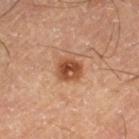The lesion was photographed on a routine skin check and not biopsied; there is no pathology result.
A lesion tile, about 15 mm wide, cut from a 3D total-body photograph.
The total-body-photography lesion software estimated a footprint of about 6.5 mm² and two-axis asymmetry of about 0.15. It also reported a border-irregularity rating of about 1.5/10 and a peripheral color-asymmetry measure near 2.5. It also reported a nevus-likeness score of about 95/100.
The patient is a male aged 58–62.
Captured under cross-polarized illumination.
About 3 mm across.
On the left thigh.